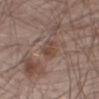About 3 mm across. The subject is a male aged approximately 65. On the right thigh. A lesion tile, about 15 mm wide, cut from a 3D total-body photograph. Imaged with white-light lighting. An algorithmic analysis of the crop reported a nevus-likeness score of about 0/100.